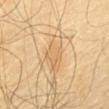Captured during whole-body skin photography for melanoma surveillance; the lesion was not biopsied. Captured under cross-polarized illumination. A male subject aged 58–62. The lesion is on the upper back. The lesion-visualizer software estimated a lesion color around L≈65 a*≈16 b*≈39 in CIELAB, about 7 CIELAB-L* units darker than the surrounding skin, and a normalized lesion–skin contrast near 4.5. And it measured a border-irregularity index near 2.5/10. The analysis additionally found a nevus-likeness score of about 0/100 and a lesion-detection confidence of about 95/100. Longest diameter approximately 5 mm. A roughly 15 mm field-of-view crop from a total-body skin photograph.